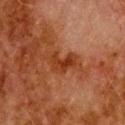biopsy status — total-body-photography surveillance lesion; no biopsy
location — the upper back
patient — male, aged 78 to 82
diameter — ≈3.5 mm
image — total-body-photography crop, ~15 mm field of view
automated lesion analysis — a border-irregularity rating of about 6/10, a within-lesion color-variation index near 2.5/10, and radial color variation of about 0.5
lighting — cross-polarized